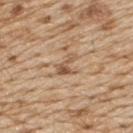Impression: Recorded during total-body skin imaging; not selected for excision or biopsy. Image and clinical context: This image is a 15 mm lesion crop taken from a total-body photograph. The tile uses white-light illumination. Measured at roughly 3.5 mm in maximum diameter. A male patient aged around 70. The lesion-visualizer software estimated an area of roughly 4.5 mm², an eccentricity of roughly 0.75, and a symmetry-axis asymmetry near 0.45. It also reported a lesion color around L≈55 a*≈18 b*≈33 in CIELAB, roughly 10 lightness units darker than nearby skin, and a normalized lesion–skin contrast near 7. The software also gave a border-irregularity rating of about 6/10, a within-lesion color-variation index near 2.5/10, and a peripheral color-asymmetry measure near 1. The analysis additionally found a classifier nevus-likeness of about 0/100. Located on the back.